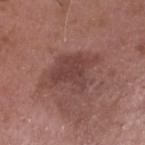notes: catalogued during a skin exam; not biopsied
location: the head or neck
image: 15 mm crop, total-body photography
patient: male, about 50 years old